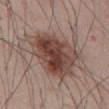This lesion was catalogued during total-body skin photography and was not selected for biopsy.